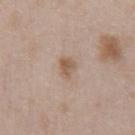This lesion was catalogued during total-body skin photography and was not selected for biopsy.
Located on the front of the torso.
A male patient, approximately 50 years of age.
A 15 mm close-up tile from a total-body photography series done for melanoma screening.
Measured at roughly 3 mm in maximum diameter.
The tile uses white-light illumination.
The lesion-visualizer software estimated a border-irregularity index near 3/10, a within-lesion color-variation index near 3/10, and peripheral color asymmetry of about 1. And it measured a detector confidence of about 100 out of 100 that the crop contains a lesion.